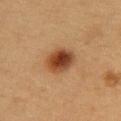follow-up: total-body-photography surveillance lesion; no biopsy | automated metrics: a lesion color around L≈41 a*≈22 b*≈33 in CIELAB and a lesion-to-skin contrast of about 11 (normalized; higher = more distinct); a color-variation rating of about 7/10 and a peripheral color-asymmetry measure near 2; a classifier nevus-likeness of about 100/100 and lesion-presence confidence of about 100/100 | anatomic site: the upper back | image: ~15 mm tile from a whole-body skin photo | patient: female, roughly 30 years of age | lesion diameter: about 4 mm.